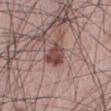follow-up: total-body-photography surveillance lesion; no biopsy.